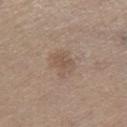follow-up = imaged on a skin check; not biopsied
patient = male, approximately 65 years of age
diameter = ~3 mm (longest diameter)
anatomic site = the leg
tile lighting = white-light
image source = ~15 mm crop, total-body skin-cancer survey